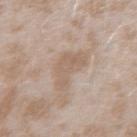This lesion was catalogued during total-body skin photography and was not selected for biopsy.
Cropped from a total-body skin-imaging series; the visible field is about 15 mm.
From the left forearm.
A female patient aged approximately 25.
Imaged with white-light lighting.